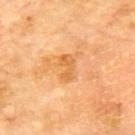notes: total-body-photography surveillance lesion; no biopsy | patient: male, in their 70s | acquisition: 15 mm crop, total-body photography | anatomic site: the upper back.